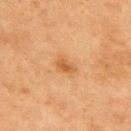biopsy status = no biopsy performed (imaged during a skin exam)
anatomic site = the upper back
lighting = cross-polarized illumination
lesion diameter = about 2.5 mm
imaging modality = 15 mm crop, total-body photography
patient = male, aged around 75